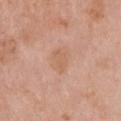Q: Is there a histopathology result?
A: no biopsy performed (imaged during a skin exam)
Q: Patient demographics?
A: female, roughly 65 years of age
Q: Illumination type?
A: white-light illumination
Q: What is the imaging modality?
A: ~15 mm tile from a whole-body skin photo
Q: What is the lesion's diameter?
A: about 3 mm
Q: What is the anatomic site?
A: the chest